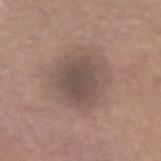| key | value |
|---|---|
| workup | catalogued during a skin exam; not biopsied |
| TBP lesion metrics | an average lesion color of about L≈49 a*≈14 b*≈21 (CIELAB) and roughly 9 lightness units darker than nearby skin; a color-variation rating of about 3.5/10 and peripheral color asymmetry of about 1; a nevus-likeness score of about 10/100 and a lesion-detection confidence of about 100/100 |
| lesion diameter | about 5.5 mm |
| illumination | white-light illumination |
| subject | male, in their mid-60s |
| anatomic site | the right forearm |
| image source | ~15 mm tile from a whole-body skin photo |